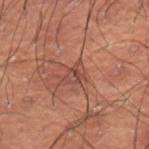Q: Is there a histopathology result?
A: total-body-photography surveillance lesion; no biopsy
Q: Illumination type?
A: cross-polarized
Q: Automated lesion metrics?
A: an average lesion color of about L≈36 a*≈20 b*≈22 (CIELAB), roughly 6 lightness units darker than nearby skin, and a normalized border contrast of about 5.5; a border-irregularity index near 3/10, a within-lesion color-variation index near 4.5/10, and a peripheral color-asymmetry measure near 1.5
Q: How large is the lesion?
A: ~2.5 mm (longest diameter)
Q: Where on the body is the lesion?
A: the lower back
Q: What is the imaging modality?
A: ~15 mm tile from a whole-body skin photo
Q: Patient demographics?
A: male, aged approximately 50